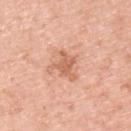biopsy_status: not biopsied; imaged during a skin examination
site: upper back
patient:
  sex: male
  age_approx: 65
lesion_size:
  long_diameter_mm_approx: 3.0
automated_metrics:
  area_mm2_approx: 6.0
  eccentricity: 0.5
  shape_asymmetry: 0.35
  color_variation_0_10: 1.5
  peripheral_color_asymmetry: 0.5
image:
  source: total-body photography crop
  field_of_view_mm: 15